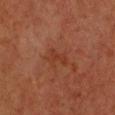The lesion was tiled from a total-body skin photograph and was not biopsied.
From the chest.
A roughly 15 mm field-of-view crop from a total-body skin photograph.
About 3 mm across.
Captured under cross-polarized illumination.
A female subject aged around 55.